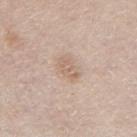workup = no biopsy performed (imaged during a skin exam) | anatomic site = the arm | acquisition = 15 mm crop, total-body photography | patient = male, about 80 years old | illumination = white-light illumination.